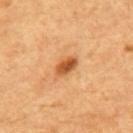Notes:
• notes: total-body-photography surveillance lesion; no biopsy
• site: the back
• image source: ~15 mm tile from a whole-body skin photo
• subject: male, aged approximately 65